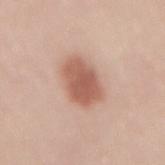The lesion was tiled from a total-body skin photograph and was not biopsied. A 15 mm close-up extracted from a 3D total-body photography capture. Captured under white-light illumination. A female patient aged 48 to 52. Longest diameter approximately 5.5 mm. The lesion is on the mid back.